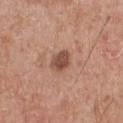The lesion is on the front of the torso.
A lesion tile, about 15 mm wide, cut from a 3D total-body photograph.
A male subject aged around 70.
The recorded lesion diameter is about 2.5 mm.
The tile uses white-light illumination.